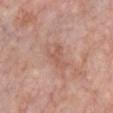Findings:
• biopsy status — catalogued during a skin exam; not biopsied
• automated lesion analysis — roughly 7 lightness units darker than nearby skin and a lesion-to-skin contrast of about 5 (normalized; higher = more distinct); a border-irregularity index near 3.5/10, a within-lesion color-variation index near 3.5/10, and peripheral color asymmetry of about 1
• body site — the chest
• acquisition — ~15 mm crop, total-body skin-cancer survey
• patient — male, aged approximately 60